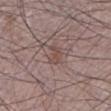This lesion was catalogued during total-body skin photography and was not selected for biopsy.